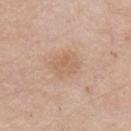Cropped from a whole-body photographic skin survey; the tile spans about 15 mm.
On the chest.
The subject is a male aged 63–67.